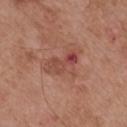Clinical impression: Recorded during total-body skin imaging; not selected for excision or biopsy. Clinical summary: The lesion's longest dimension is about 5 mm. A male patient, aged approximately 55. From the mid back. A close-up tile cropped from a whole-body skin photograph, about 15 mm across. Imaged with white-light lighting.